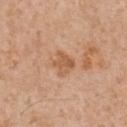The lesion was photographed on a routine skin check and not biopsied; there is no pathology result.